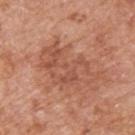workup — imaged on a skin check; not biopsied
patient — male, in their mid-60s
site — the upper back
imaging modality — ~15 mm tile from a whole-body skin photo